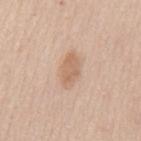Q: Was this lesion biopsied?
A: no biopsy performed (imaged during a skin exam)
Q: Who is the patient?
A: female, in their mid-40s
Q: Lesion location?
A: the mid back
Q: What is the imaging modality?
A: total-body-photography crop, ~15 mm field of view